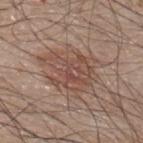Part of a total-body skin-imaging series; this lesion was reviewed on a skin check and was not flagged for biopsy. A region of skin cropped from a whole-body photographic capture, roughly 15 mm wide. On the upper back. A male subject in their mid- to late 40s. Measured at roughly 5.5 mm in maximum diameter.